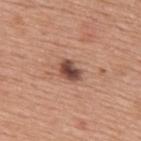Findings:
* notes: imaged on a skin check; not biopsied
* site: the upper back
* subject: male, approximately 75 years of age
* acquisition: total-body-photography crop, ~15 mm field of view